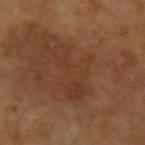Impression:
Part of a total-body skin-imaging series; this lesion was reviewed on a skin check and was not flagged for biopsy.
Clinical summary:
The tile uses cross-polarized illumination. The lesion is located on the left upper arm. A male patient, in their mid- to late 60s. Automated tile analysis of the lesion measured a border-irregularity index near 5.5/10, internal color variation of about 4.5 on a 0–10 scale, and peripheral color asymmetry of about 1.5. The analysis additionally found a lesion-detection confidence of about 95/100. Cropped from a total-body skin-imaging series; the visible field is about 15 mm. The lesion's longest dimension is about 16.5 mm.